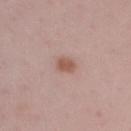Captured during whole-body skin photography for melanoma surveillance; the lesion was not biopsied. The total-body-photography lesion software estimated a lesion area of about 3.5 mm² and two-axis asymmetry of about 0.25. The analysis additionally found a classifier nevus-likeness of about 95/100 and lesion-presence confidence of about 100/100. A 15 mm close-up extracted from a 3D total-body photography capture. A female patient aged 43–47. From the right upper arm. This is a white-light tile.